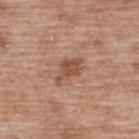notes — imaged on a skin check; not biopsied | anatomic site — the upper back | automated lesion analysis — an area of roughly 5.5 mm² and a shape-asymmetry score of about 0.35 (0 = symmetric); a lesion–skin lightness drop of about 10 and a lesion-to-skin contrast of about 7 (normalized; higher = more distinct) | image source — 15 mm crop, total-body photography | lighting — white-light | diameter — about 3.5 mm | patient — male, approximately 50 years of age.